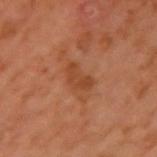{"biopsy_status": "not biopsied; imaged during a skin examination", "image": {"source": "total-body photography crop", "field_of_view_mm": 15}, "automated_metrics": {"cielab_L": 41, "cielab_a": 24, "cielab_b": 34, "vs_skin_contrast_norm": 6.0, "nevus_likeness_0_100": 0, "lesion_detection_confidence_0_100": 100}, "patient": {"sex": "male", "age_approx": 60}, "site": "left upper arm", "lighting": "cross-polarized", "lesion_size": {"long_diameter_mm_approx": 3.5}}